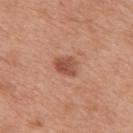Assessment: This lesion was catalogued during total-body skin photography and was not selected for biopsy. Acquisition and patient details: Captured under white-light illumination. A roughly 15 mm field-of-view crop from a total-body skin photograph. The patient is a male approximately 70 years of age. The lesion's longest dimension is about 3 mm. The lesion is on the mid back.